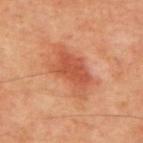Part of a total-body skin-imaging series; this lesion was reviewed on a skin check and was not flagged for biopsy. About 6.5 mm across. A male subject, aged 58–62. Automated image analysis of the tile measured a lesion area of about 16 mm², an eccentricity of roughly 0.85, and two-axis asymmetry of about 0.35. And it measured a nevus-likeness score of about 85/100. On the chest. A 15 mm close-up extracted from a 3D total-body photography capture. The tile uses cross-polarized illumination.